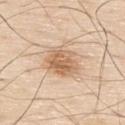biopsy_status: not biopsied; imaged during a skin examination
image:
  source: total-body photography crop
  field_of_view_mm: 15
lighting: white-light
patient:
  sex: male
  age_approx: 80
lesion_size:
  long_diameter_mm_approx: 4.0
automated_metrics:
  cielab_L: 64
  cielab_a: 18
  cielab_b: 34
  vs_skin_darker_L: 12.0
  vs_skin_contrast_norm: 7.5
  border_irregularity_0_10: 2.0
site: upper back